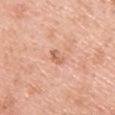Assessment: The lesion was tiled from a total-body skin photograph and was not biopsied. Image and clinical context: Longest diameter approximately 2.5 mm. On the upper back. A male subject, approximately 60 years of age. This is a white-light tile. A 15 mm close-up extracted from a 3D total-body photography capture.